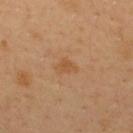biopsy_status: not biopsied; imaged during a skin examination
image:
  source: total-body photography crop
  field_of_view_mm: 15
patient:
  sex: male
  age_approx: 40
site: upper back
lesion_size:
  long_diameter_mm_approx: 3.0
lighting: cross-polarized
automated_metrics:
  vs_skin_darker_L: 6.0
  vs_skin_contrast_norm: 5.5
  border_irregularity_0_10: 4.0
  nevus_likeness_0_100: 0
  lesion_detection_confidence_0_100: 100